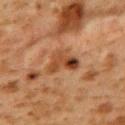notes — catalogued during a skin exam; not biopsied | diameter — about 4 mm | image — ~15 mm crop, total-body skin-cancer survey | anatomic site — the upper back | illumination — cross-polarized illumination | subject — female, aged 38–42.